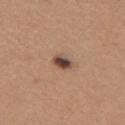follow-up: no biopsy performed (imaged during a skin exam)
location: the upper back
lesion diameter: about 2.5 mm
image: total-body-photography crop, ~15 mm field of view
patient: female, aged 28–32